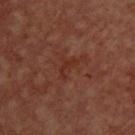follow-up=imaged on a skin check; not biopsied | illumination=cross-polarized | patient=female, aged approximately 45 | imaging modality=~15 mm crop, total-body skin-cancer survey | body site=the back.